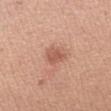{
  "biopsy_status": "not biopsied; imaged during a skin examination",
  "patient": {
    "sex": "female",
    "age_approx": 30
  },
  "lighting": "white-light",
  "image": {
    "source": "total-body photography crop",
    "field_of_view_mm": 15
  },
  "site": "left upper arm",
  "lesion_size": {
    "long_diameter_mm_approx": 2.5
  }
}